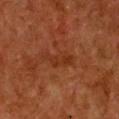This lesion was catalogued during total-body skin photography and was not selected for biopsy. A female patient aged around 50. This image is a 15 mm lesion crop taken from a total-body photograph. From the chest. Imaged with cross-polarized lighting. Longest diameter approximately 4 mm. An algorithmic analysis of the crop reported roughly 5 lightness units darker than nearby skin and a lesion-to-skin contrast of about 5.5 (normalized; higher = more distinct). It also reported an automated nevus-likeness rating near 0 out of 100 and lesion-presence confidence of about 100/100.